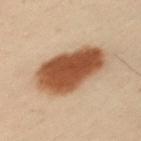follow-up — catalogued during a skin exam; not biopsied
patient — male, aged approximately 55
location — the left upper arm
imaging modality — ~15 mm tile from a whole-body skin photo